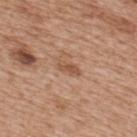  biopsy_status: not biopsied; imaged during a skin examination
  site: upper back
  automated_metrics:
    area_mm2_approx: 3.0
    shape_asymmetry: 0.3
    border_irregularity_0_10: 3.5
    peripheral_color_asymmetry: 0.0
  patient:
    sex: male
    age_approx: 65
  image:
    source: total-body photography crop
    field_of_view_mm: 15
  lesion_size:
    long_diameter_mm_approx: 3.0